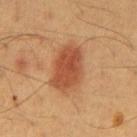Notes:
– follow-up · catalogued during a skin exam; not biopsied
– lighting · cross-polarized
– subject · male, aged 58 to 62
– body site · the abdomen
– lesion diameter · ~6 mm (longest diameter)
– image source · ~15 mm tile from a whole-body skin photo